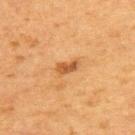| feature | finding |
|---|---|
| notes | total-body-photography surveillance lesion; no biopsy |
| body site | the upper back |
| lesion size | ≈3 mm |
| patient | female, aged approximately 50 |
| image | 15 mm crop, total-body photography |